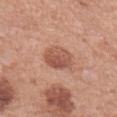The lesion was tiled from a total-body skin photograph and was not biopsied.
The tile uses white-light illumination.
A 15 mm close-up tile from a total-body photography series done for melanoma screening.
Located on the left forearm.
Automated tile analysis of the lesion measured a mean CIELAB color near L≈54 a*≈25 b*≈29 and a normalized lesion–skin contrast near 7. And it measured border irregularity of about 1.5 on a 0–10 scale, a within-lesion color-variation index near 4.5/10, and peripheral color asymmetry of about 1.5. The analysis additionally found a lesion-detection confidence of about 100/100.
A female patient, in their 60s.
About 3.5 mm across.